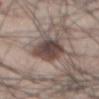This lesion was catalogued during total-body skin photography and was not selected for biopsy. Approximately 6.5 mm at its widest. A male subject, aged 43–47. The lesion is located on the abdomen. A region of skin cropped from a whole-body photographic capture, roughly 15 mm wide.